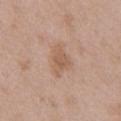follow-up: no biopsy performed (imaged during a skin exam); location: the chest; acquisition: ~15 mm tile from a whole-body skin photo; patient: male, aged around 50.